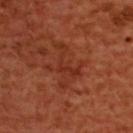workup = imaged on a skin check; not biopsied | location = the upper back | imaging modality = 15 mm crop, total-body photography | patient = female, in their mid- to late 50s | lesion diameter = about 5 mm | tile lighting = cross-polarized illumination.